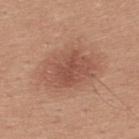notes: imaged on a skin check; not biopsied
tile lighting: white-light illumination
location: the upper back
diameter: ~6 mm (longest diameter)
image: 15 mm crop, total-body photography
subject: male, in their 30s